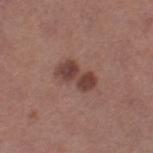Notes:
* follow-up: catalogued during a skin exam; not biopsied
* patient: female, in their 50s
* imaging modality: ~15 mm tile from a whole-body skin photo
* diameter: ≈4.5 mm
* tile lighting: white-light
* location: the left thigh